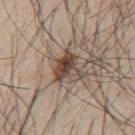This lesion was catalogued during total-body skin photography and was not selected for biopsy.